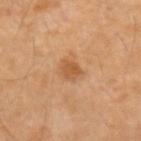{"biopsy_status": "not biopsied; imaged during a skin examination", "patient": {"sex": "female", "age_approx": 60}, "site": "arm", "image": {"source": "total-body photography crop", "field_of_view_mm": 15}}